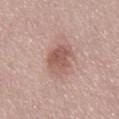Q: Automated lesion metrics?
A: a lesion area of about 10 mm², an outline eccentricity of about 0.75 (0 = round, 1 = elongated), and two-axis asymmetry of about 0.2; a mean CIELAB color near L≈56 a*≈21 b*≈24 and a normalized lesion–skin contrast near 7.5; border irregularity of about 2.5 on a 0–10 scale, a color-variation rating of about 4/10, and peripheral color asymmetry of about 1.5
Q: How large is the lesion?
A: ~4 mm (longest diameter)
Q: Lesion location?
A: the front of the torso
Q: What kind of image is this?
A: total-body-photography crop, ~15 mm field of view
Q: Patient demographics?
A: male, aged around 55